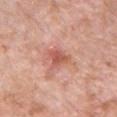workup = total-body-photography surveillance lesion; no biopsy | anatomic site = the front of the torso | image = ~15 mm tile from a whole-body skin photo | subject = male, aged approximately 50.